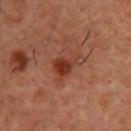{"biopsy_status": "not biopsied; imaged during a skin examination", "image": {"source": "total-body photography crop", "field_of_view_mm": 15}, "lesion_size": {"long_diameter_mm_approx": 4.0}, "automated_metrics": {"area_mm2_approx": 6.5, "eccentricity": 0.8, "shape_asymmetry": 0.5, "cielab_L": 37, "cielab_a": 26, "cielab_b": 29, "vs_skin_darker_L": 9.0, "vs_skin_contrast_norm": 8.0}, "site": "upper back", "lighting": "cross-polarized", "patient": {"sex": "male", "age_approx": 55}}